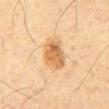Clinical impression:
The lesion was photographed on a routine skin check and not biopsied; there is no pathology result.
Acquisition and patient details:
This is a cross-polarized tile. Located on the abdomen. A roughly 15 mm field-of-view crop from a total-body skin photograph. A male subject roughly 60 years of age.